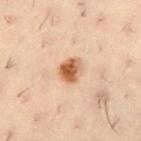Approximately 3 mm at its widest. Imaged with cross-polarized lighting. Located on the left thigh. Automated tile analysis of the lesion measured a nevus-likeness score of about 100/100 and lesion-presence confidence of about 100/100. A male patient, in their 30s. A 15 mm close-up extracted from a 3D total-body photography capture.